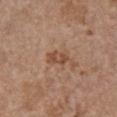Imaged during a routine full-body skin examination; the lesion was not biopsied and no histopathology is available.
Automated image analysis of the tile measured a lesion area of about 4.5 mm², an eccentricity of roughly 0.8, and two-axis asymmetry of about 0.25. And it measured a lesion color around L≈49 a*≈20 b*≈31 in CIELAB, a lesion–skin lightness drop of about 9, and a normalized lesion–skin contrast near 7. And it measured a border-irregularity rating of about 2.5/10 and a within-lesion color-variation index near 3/10.
Located on the chest.
A female patient, about 65 years old.
A region of skin cropped from a whole-body photographic capture, roughly 15 mm wide.